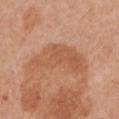Impression:
Imaged during a routine full-body skin examination; the lesion was not biopsied and no histopathology is available.
Context:
A female subject, aged approximately 55. A region of skin cropped from a whole-body photographic capture, roughly 15 mm wide. The total-body-photography lesion software estimated a lesion color around L≈56 a*≈24 b*≈34 in CIELAB and a normalized lesion–skin contrast near 5.5. The analysis additionally found border irregularity of about 6 on a 0–10 scale and a within-lesion color-variation index near 2.5/10. Measured at roughly 7.5 mm in maximum diameter. On the chest.